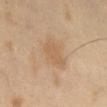{"biopsy_status": "not biopsied; imaged during a skin examination", "image": {"source": "total-body photography crop", "field_of_view_mm": 15}, "patient": {"sex": "male", "age_approx": 65}}